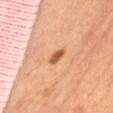Clinical impression:
Recorded during total-body skin imaging; not selected for excision or biopsy.
Context:
A female patient. On the front of the torso. Captured under cross-polarized illumination. Cropped from a total-body skin-imaging series; the visible field is about 15 mm.